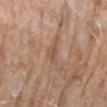workup = catalogued during a skin exam; not biopsied | image source = 15 mm crop, total-body photography | anatomic site = the arm | automated metrics = a footprint of about 4 mm²; a border-irregularity rating of about 4/10, a color-variation rating of about 2/10, and radial color variation of about 0.5 | subject = female, roughly 85 years of age or older | illumination = white-light | lesion diameter = about 3 mm.